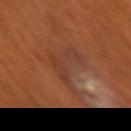Part of a total-body skin-imaging series; this lesion was reviewed on a skin check and was not flagged for biopsy.
A female subject aged approximately 80.
The lesion-visualizer software estimated a footprint of about 17 mm² and an eccentricity of roughly 0.85. The software also gave a mean CIELAB color near L≈28 a*≈17 b*≈23, about 4 CIELAB-L* units darker than the surrounding skin, and a lesion-to-skin contrast of about 5 (normalized; higher = more distinct). And it measured lesion-presence confidence of about 80/100.
The tile uses cross-polarized illumination.
Cropped from a total-body skin-imaging series; the visible field is about 15 mm.
On the left upper arm.